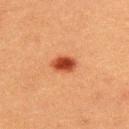notes: no biopsy performed (imaged during a skin exam) | image-analysis metrics: an area of roughly 5.5 mm²; border irregularity of about 1.5 on a 0–10 scale, a within-lesion color-variation index near 4/10, and radial color variation of about 1.5 | anatomic site: the upper back | tile lighting: cross-polarized illumination | image: total-body-photography crop, ~15 mm field of view | patient: male, aged 38–42 | size: ~3 mm (longest diameter).